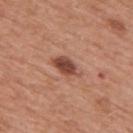Assessment: This lesion was catalogued during total-body skin photography and was not selected for biopsy. Clinical summary: A region of skin cropped from a whole-body photographic capture, roughly 15 mm wide. This is a white-light tile. A male patient in their 70s. The lesion is located on the mid back.